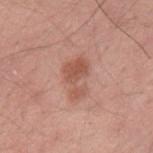{
  "biopsy_status": "not biopsied; imaged during a skin examination",
  "lighting": "white-light",
  "patient": {
    "sex": "male",
    "age_approx": 25
  },
  "automated_metrics": {
    "eccentricity": 0.85,
    "shape_asymmetry": 0.5,
    "cielab_L": 54,
    "cielab_a": 24,
    "cielab_b": 29,
    "vs_skin_contrast_norm": 6.5
  },
  "lesion_size": {
    "long_diameter_mm_approx": 4.5
  },
  "site": "left upper arm",
  "image": {
    "source": "total-body photography crop",
    "field_of_view_mm": 15
  }
}